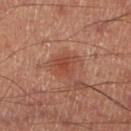Recorded during total-body skin imaging; not selected for excision or biopsy.